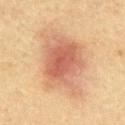<tbp_lesion>
<biopsy_status>not biopsied; imaged during a skin examination</biopsy_status>
<lighting>cross-polarized</lighting>
<lesion_size>
  <long_diameter_mm_approx>7.5</long_diameter_mm_approx>
</lesion_size>
<patient>
  <sex>male</sex>
  <age_approx>60</age_approx>
</patient>
<image>
  <source>total-body photography crop</source>
  <field_of_view_mm>15</field_of_view_mm>
</image>
</tbp_lesion>